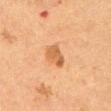This lesion was catalogued during total-body skin photography and was not selected for biopsy. The patient is a female aged around 50. The tile uses cross-polarized illumination. This image is a 15 mm lesion crop taken from a total-body photograph. An algorithmic analysis of the crop reported a lesion area of about 6 mm² and an outline eccentricity of about 0.75 (0 = round, 1 = elongated). And it measured an average lesion color of about L≈50 a*≈21 b*≈35 (CIELAB), about 9 CIELAB-L* units darker than the surrounding skin, and a normalized lesion–skin contrast near 7. It also reported a border-irregularity index near 2/10, a within-lesion color-variation index near 2.5/10, and radial color variation of about 1. Approximately 3.5 mm at its widest. The lesion is on the abdomen.